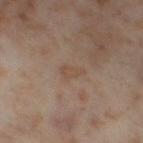The lesion was photographed on a routine skin check and not biopsied; there is no pathology result.
A roughly 15 mm field-of-view crop from a total-body skin photograph.
On the right thigh.
The subject is a female approximately 55 years of age.
Longest diameter approximately 3 mm.
Imaged with cross-polarized lighting.